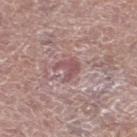Findings:
* notes · imaged on a skin check; not biopsied
* site · the left lower leg
* subject · male, roughly 65 years of age
* image source · ~15 mm crop, total-body skin-cancer survey